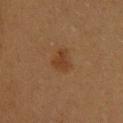<case>
  <biopsy_status>not biopsied; imaged during a skin examination</biopsy_status>
  <site>upper back</site>
  <lighting>cross-polarized</lighting>
  <automated_metrics>
    <cielab_L>34</cielab_L>
    <cielab_a>18</cielab_a>
    <cielab_b>30</cielab_b>
    <vs_skin_darker_L>6.0</vs_skin_darker_L>
    <vs_skin_contrast_norm>6.5</vs_skin_contrast_norm>
    <nevus_likeness_0_100>75</nevus_likeness_0_100>
  </automated_metrics>
  <image>
    <source>total-body photography crop</source>
    <field_of_view_mm>15</field_of_view_mm>
  </image>
  <lesion_size>
    <long_diameter_mm_approx>3.0</long_diameter_mm_approx>
  </lesion_size>
  <patient>
    <sex>female</sex>
    <age_approx>20</age_approx>
  </patient>
</case>